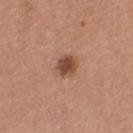The lesion was photographed on a routine skin check and not biopsied; there is no pathology result.
The patient is a female roughly 60 years of age.
Imaged with white-light lighting.
On the left thigh.
This image is a 15 mm lesion crop taken from a total-body photograph.
The lesion's longest dimension is about 2.5 mm.